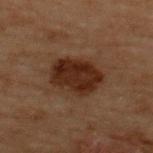follow-up — catalogued during a skin exam; not biopsied | imaging modality — ~15 mm tile from a whole-body skin photo | patient — male, about 70 years old | automated lesion analysis — a mean CIELAB color near L≈20 a*≈16 b*≈21, a lesion–skin lightness drop of about 9, and a lesion-to-skin contrast of about 11 (normalized; higher = more distinct); a border-irregularity index near 1.5/10 and a within-lesion color-variation index near 3.5/10; a classifier nevus-likeness of about 65/100 and lesion-presence confidence of about 100/100 | illumination — cross-polarized illumination | lesion size — ≈5 mm | body site — the upper back.